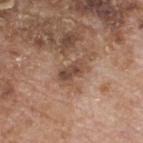Impression:
Imaged during a routine full-body skin examination; the lesion was not biopsied and no histopathology is available.
Background:
The patient is a male aged around 80. Captured under white-light illumination. This image is a 15 mm lesion crop taken from a total-body photograph. Located on the upper back. The lesion's longest dimension is about 3 mm.